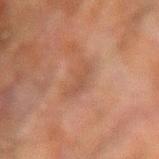Assessment:
Part of a total-body skin-imaging series; this lesion was reviewed on a skin check and was not flagged for biopsy.
Context:
Located on the left arm. The recorded lesion diameter is about 3.5 mm. Imaged with cross-polarized lighting. A roughly 15 mm field-of-view crop from a total-body skin photograph. A male subject aged 58 to 62.